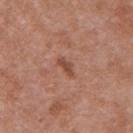Part of a total-body skin-imaging series; this lesion was reviewed on a skin check and was not flagged for biopsy.
A close-up tile cropped from a whole-body skin photograph, about 15 mm across.
Located on the left upper arm.
The patient is a female aged around 40.
Captured under white-light illumination.